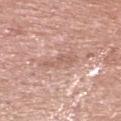notes — imaged on a skin check; not biopsied | patient — male, aged approximately 65 | lighting — white-light illumination | image source — 15 mm crop, total-body photography | location — the head or neck.